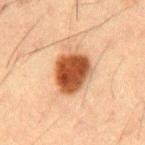<case>
  <biopsy_status>not biopsied; imaged during a skin examination</biopsy_status>
  <automated_metrics>
    <area_mm2_approx>15.0</area_mm2_approx>
    <eccentricity>0.6</eccentricity>
    <shape_asymmetry>0.2</shape_asymmetry>
    <cielab_L>41</cielab_L>
    <cielab_a>22</cielab_a>
    <cielab_b>32</cielab_b>
    <vs_skin_darker_L>17.0</vs_skin_darker_L>
    <vs_skin_contrast_norm>13.0</vs_skin_contrast_norm>
    <nevus_likeness_0_100>100</nevus_likeness_0_100>
    <lesion_detection_confidence_0_100>100</lesion_detection_confidence_0_100>
  </automated_metrics>
  <site>mid back</site>
  <patient>
    <sex>male</sex>
    <age_approx>50</age_approx>
  </patient>
  <lighting>cross-polarized</lighting>
  <lesion_size>
    <long_diameter_mm_approx>5.0</long_diameter_mm_approx>
  </lesion_size>
  <image>
    <source>total-body photography crop</source>
    <field_of_view_mm>15</field_of_view_mm>
  </image>
</case>